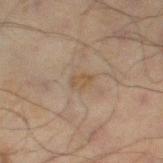{
  "biopsy_status": "not biopsied; imaged during a skin examination"
}